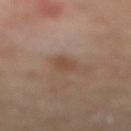  biopsy_status: not biopsied; imaged during a skin examination
  patient:
    sex: female
    age_approx: 75
  site: left lower leg
  image:
    source: total-body photography crop
    field_of_view_mm: 15